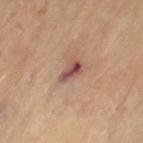biopsy status = catalogued during a skin exam; not biopsied.